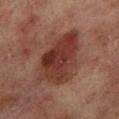This image is a 15 mm lesion crop taken from a total-body photograph.
The total-body-photography lesion software estimated two-axis asymmetry of about 0.3. The software also gave border irregularity of about 3.5 on a 0–10 scale, a color-variation rating of about 4.5/10, and radial color variation of about 1.5. The software also gave a nevus-likeness score of about 30/100 and a detector confidence of about 100 out of 100 that the crop contains a lesion.
Approximately 7 mm at its widest.
A male patient roughly 70 years of age.
From the leg.